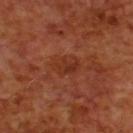No biopsy was performed on this lesion — it was imaged during a full skin examination and was not determined to be concerning. On the upper back. The recorded lesion diameter is about 3.5 mm. The subject is a male aged around 70. The lesion-visualizer software estimated an automated nevus-likeness rating near 0 out of 100 and lesion-presence confidence of about 100/100. Imaged with cross-polarized lighting. Cropped from a total-body skin-imaging series; the visible field is about 15 mm.